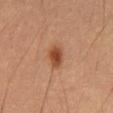| key | value |
|---|---|
| workup | catalogued during a skin exam; not biopsied |
| anatomic site | the mid back |
| tile lighting | cross-polarized illumination |
| automated lesion analysis | an average lesion color of about L≈40 a*≈21 b*≈30 (CIELAB) and a normalized lesion–skin contrast near 9; a border-irregularity rating of about 2/10, a within-lesion color-variation index near 3/10, and radial color variation of about 0.5; a classifier nevus-likeness of about 100/100 and a lesion-detection confidence of about 100/100 |
| diameter | about 3.5 mm |
| acquisition | 15 mm crop, total-body photography |
| subject | male, aged 53–57 |